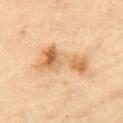workup = catalogued during a skin exam; not biopsied
anatomic site = the left thigh
image = total-body-photography crop, ~15 mm field of view
lesion size = ~6.5 mm (longest diameter)
patient = female, aged around 70
illumination = cross-polarized illumination
TBP lesion metrics = an eccentricity of roughly 0.9 and two-axis asymmetry of about 0.55; an automated nevus-likeness rating near 65 out of 100 and a lesion-detection confidence of about 100/100